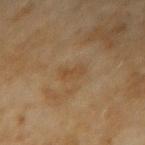Imaged during a routine full-body skin examination; the lesion was not biopsied and no histopathology is available.
A female subject, aged around 60.
A region of skin cropped from a whole-body photographic capture, roughly 15 mm wide.
From the left forearm.
Automated tile analysis of the lesion measured a lesion area of about 3 mm² and a shape-asymmetry score of about 0.35 (0 = symmetric). The software also gave a border-irregularity rating of about 3.5/10, internal color variation of about 0.5 on a 0–10 scale, and radial color variation of about 0. The analysis additionally found an automated nevus-likeness rating near 0 out of 100 and lesion-presence confidence of about 100/100.